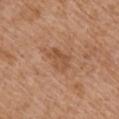notes: imaged on a skin check; not biopsied
lighting: white-light
subject: male, aged around 75
size: ~3.5 mm (longest diameter)
anatomic site: the right upper arm
TBP lesion metrics: an area of roughly 6.5 mm², a shape eccentricity near 0.75, and a shape-asymmetry score of about 0.3 (0 = symmetric); a border-irregularity index near 3/10, internal color variation of about 3 on a 0–10 scale, and a peripheral color-asymmetry measure near 1.5
image: 15 mm crop, total-body photography